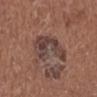Clinical summary: The tile uses white-light illumination. A female patient, aged 48 to 52. A 15 mm close-up extracted from a 3D total-body photography capture. On the left lower leg. Automated tile analysis of the lesion measured a lesion area of about 13 mm², an eccentricity of roughly 0.85, and two-axis asymmetry of about 0.45. The analysis additionally found a border-irregularity index near 6.5/10, a within-lesion color-variation index near 5/10, and a peripheral color-asymmetry measure near 1.5.